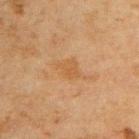A male subject, approximately 45 years of age. The lesion is on the back. The total-body-photography lesion software estimated an average lesion color of about L≈46 a*≈18 b*≈34 (CIELAB), a lesion–skin lightness drop of about 5, and a normalized border contrast of about 5.5. The software also gave a detector confidence of about 100 out of 100 that the crop contains a lesion. A 15 mm close-up tile from a total-body photography series done for melanoma screening. Captured under cross-polarized illumination.